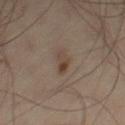• follow-up · imaged on a skin check; not biopsied
• image source · ~15 mm crop, total-body skin-cancer survey
• automated lesion analysis · a footprint of about 3 mm², a shape eccentricity near 0.9, and a shape-asymmetry score of about 0.4 (0 = symmetric); a border-irregularity index near 4/10, a within-lesion color-variation index near 1/10, and peripheral color asymmetry of about 0
• site · the left leg
• subject · male, aged around 50
• size · about 2.5 mm